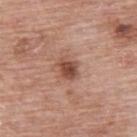{"biopsy_status": "not biopsied; imaged during a skin examination", "site": "upper back", "lighting": "white-light", "patient": {"sex": "female", "age_approx": 60}, "image": {"source": "total-body photography crop", "field_of_view_mm": 15}, "automated_metrics": {"area_mm2_approx": 5.0, "eccentricity": 0.6, "shape_asymmetry": 0.2, "color_variation_0_10": 3.5, "peripheral_color_asymmetry": 1.0, "nevus_likeness_0_100": 85, "lesion_detection_confidence_0_100": 100}}